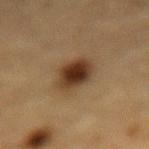automated metrics = a lesion color around L≈33 a*≈15 b*≈27 in CIELAB and a normalized border contrast of about 11; border irregularity of about 2 on a 0–10 scale and peripheral color asymmetry of about 1.5; a classifier nevus-likeness of about 100/100 and a detector confidence of about 100 out of 100 that the crop contains a lesion
illumination = cross-polarized
body site = the lower back
image source = ~15 mm tile from a whole-body skin photo
patient = male, about 85 years old or older
size = ≈5 mm